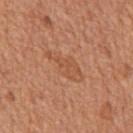| feature | finding |
|---|---|
| workup | catalogued during a skin exam; not biopsied |
| automated metrics | a footprint of about 7 mm² and an outline eccentricity of about 0.95 (0 = round, 1 = elongated); about 6 CIELAB-L* units darker than the surrounding skin and a normalized lesion–skin contrast near 4.5 |
| size | ~5 mm (longest diameter) |
| anatomic site | the mid back |
| illumination | white-light |
| acquisition | ~15 mm crop, total-body skin-cancer survey |
| patient | male, aged around 65 |